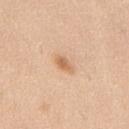Impression:
No biopsy was performed on this lesion — it was imaged during a full skin examination and was not determined to be concerning.
Image and clinical context:
A female patient roughly 55 years of age. From the mid back. A close-up tile cropped from a whole-body skin photograph, about 15 mm across.